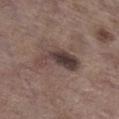workup = catalogued during a skin exam; not biopsied
tile lighting = white-light illumination
patient = male, aged 68–72
anatomic site = the right lower leg
acquisition = total-body-photography crop, ~15 mm field of view